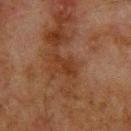Case summary:
* notes: total-body-photography surveillance lesion; no biopsy
* imaging modality: total-body-photography crop, ~15 mm field of view
* site: the upper back
* subject: male, aged around 65